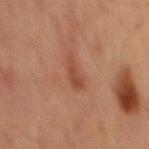lesion diameter: about 3.5 mm
tile lighting: cross-polarized
location: the front of the torso
imaging modality: ~15 mm tile from a whole-body skin photo
automated lesion analysis: a lesion area of about 4 mm² and a shape eccentricity near 0.95; border irregularity of about 4.5 on a 0–10 scale, a within-lesion color-variation index near 0.5/10, and peripheral color asymmetry of about 0
subject: male, in their mid- to late 60s